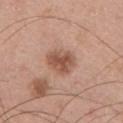follow-up = catalogued during a skin exam; not biopsied
location = the front of the torso
illumination = white-light illumination
diameter = ≈4 mm
acquisition = ~15 mm tile from a whole-body skin photo
subject = male, approximately 30 years of age